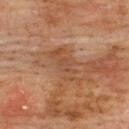No biopsy was performed on this lesion — it was imaged during a full skin examination and was not determined to be concerning.
The subject is a male roughly 75 years of age.
The total-body-photography lesion software estimated a lesion color around L≈40 a*≈18 b*≈28 in CIELAB, roughly 6 lightness units darker than nearby skin, and a normalized lesion–skin contrast near 5. And it measured a classifier nevus-likeness of about 0/100 and a lesion-detection confidence of about 80/100.
This image is a 15 mm lesion crop taken from a total-body photograph.
Located on the upper back.
Captured under cross-polarized illumination.